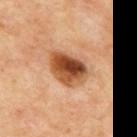Clinical impression:
Captured during whole-body skin photography for melanoma surveillance; the lesion was not biopsied.
Context:
A patient approximately 65 years of age. Cropped from a whole-body photographic skin survey; the tile spans about 15 mm. The total-body-photography lesion software estimated an area of roughly 13 mm², a shape eccentricity near 0.75, and two-axis asymmetry of about 0.15. And it measured a border-irregularity index near 1.5/10, a within-lesion color-variation index near 10/10, and a peripheral color-asymmetry measure near 3.5. The lesion is on the mid back. The lesion's longest dimension is about 5 mm.